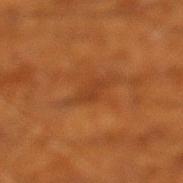* biopsy status — total-body-photography surveillance lesion; no biopsy
* image source — 15 mm crop, total-body photography
* patient — male, approximately 60 years of age
* site — the left lower leg